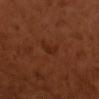Q: Was this lesion biopsied?
A: imaged on a skin check; not biopsied
Q: How was this image acquired?
A: 15 mm crop, total-body photography
Q: Illumination type?
A: cross-polarized
Q: Who is the patient?
A: male, aged 48–52
Q: Where on the body is the lesion?
A: the right upper arm
Q: Lesion size?
A: ~2.5 mm (longest diameter)